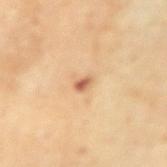The lesion was photographed on a routine skin check and not biopsied; there is no pathology result. Captured under cross-polarized illumination. Automated image analysis of the tile measured a lesion-to-skin contrast of about 8.5 (normalized; higher = more distinct). It also reported border irregularity of about 2.5 on a 0–10 scale and a color-variation rating of about 0.5/10. The analysis additionally found a nevus-likeness score of about 25/100 and a detector confidence of about 100 out of 100 that the crop contains a lesion. Approximately 2 mm at its widest. The subject is a male aged 53 to 57. The lesion is located on the right forearm. This image is a 15 mm lesion crop taken from a total-body photograph.